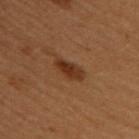Clinical impression:
The lesion was photographed on a routine skin check and not biopsied; there is no pathology result.
Image and clinical context:
The patient is a female aged 48–52. The lesion-visualizer software estimated a footprint of about 4.5 mm², an outline eccentricity of about 0.85 (0 = round, 1 = elongated), and two-axis asymmetry of about 0.25. And it measured a border-irregularity index near 2.5/10 and a within-lesion color-variation index near 3/10. Located on the back. A 15 mm close-up extracted from a 3D total-body photography capture. The tile uses cross-polarized illumination.